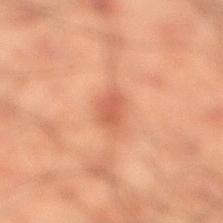biopsy status — no biopsy performed (imaged during a skin exam)
image source — total-body-photography crop, ~15 mm field of view
tile lighting — cross-polarized
body site — the right lower leg
patient — male, aged approximately 50